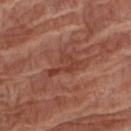Q: Was a biopsy performed?
A: imaged on a skin check; not biopsied
Q: What is the anatomic site?
A: the right forearm
Q: What lighting was used for the tile?
A: white-light illumination
Q: What is the imaging modality?
A: ~15 mm tile from a whole-body skin photo
Q: Patient demographics?
A: female, in their 80s